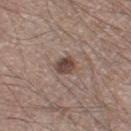Impression:
Imaged during a routine full-body skin examination; the lesion was not biopsied and no histopathology is available.
Background:
The patient is a male aged around 65. From the left thigh. Automated image analysis of the tile measured an eccentricity of roughly 0.65 and a shape-asymmetry score of about 0.2 (0 = symmetric). And it measured a lesion-to-skin contrast of about 9.5 (normalized; higher = more distinct). And it measured a detector confidence of about 100 out of 100 that the crop contains a lesion. A lesion tile, about 15 mm wide, cut from a 3D total-body photograph. Measured at roughly 2.5 mm in maximum diameter. The tile uses white-light illumination.